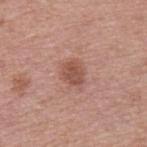This lesion was catalogued during total-body skin photography and was not selected for biopsy.
From the upper back.
A male subject, aged 38 to 42.
A 15 mm close-up extracted from a 3D total-body photography capture.
Imaged with white-light lighting.